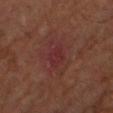biopsy status: total-body-photography surveillance lesion; no biopsy
site: the right upper arm
patient: male, aged approximately 65
image-analysis metrics: an area of roughly 5 mm² and two-axis asymmetry of about 0.25; an automated nevus-likeness rating near 0 out of 100 and a lesion-detection confidence of about 95/100
image source: 15 mm crop, total-body photography
lighting: cross-polarized